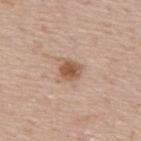| field | value |
|---|---|
| workup | imaged on a skin check; not biopsied |
| patient | male, approximately 75 years of age |
| tile lighting | white-light illumination |
| imaging modality | ~15 mm crop, total-body skin-cancer survey |
| location | the mid back |
| lesion diameter | ~2.5 mm (longest diameter) |